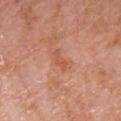| key | value |
|---|---|
| lesion diameter | ~3 mm (longest diameter) |
| illumination | white-light illumination |
| body site | the front of the torso |
| patient | male, aged around 75 |
| image | total-body-photography crop, ~15 mm field of view |
| automated metrics | a border-irregularity index near 4/10, a color-variation rating of about 2.5/10, and a peripheral color-asymmetry measure near 1; a lesion-detection confidence of about 100/100 |